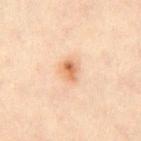Case summary:
• image-analysis metrics · a within-lesion color-variation index near 6.5/10 and radial color variation of about 2
• acquisition · ~15 mm tile from a whole-body skin photo
• patient · male, in their mid- to late 30s
• tile lighting · cross-polarized illumination
• location · the abdomen
• size · ≈2.5 mm